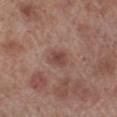This lesion was catalogued during total-body skin photography and was not selected for biopsy. The subject is a male aged 68–72. This image is a 15 mm lesion crop taken from a total-body photograph. Located on the left lower leg.